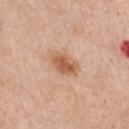Impression: No biopsy was performed on this lesion — it was imaged during a full skin examination and was not determined to be concerning. Background: The lesion-visualizer software estimated a shape eccentricity near 0.8 and a shape-asymmetry score of about 0.2 (0 = symmetric). The analysis additionally found a lesion color around L≈59 a*≈23 b*≈34 in CIELAB, roughly 11 lightness units darker than nearby skin, and a lesion-to-skin contrast of about 8 (normalized; higher = more distinct). It also reported a border-irregularity index near 2/10, a within-lesion color-variation index near 3/10, and a peripheral color-asymmetry measure near 1. The recorded lesion diameter is about 4 mm. A male subject, aged approximately 60. Located on the chest. Imaged with white-light lighting. A 15 mm close-up extracted from a 3D total-body photography capture.